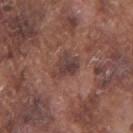biopsy_status: not biopsied; imaged during a skin examination
patient:
  sex: male
  age_approx: 75
lighting: white-light
image:
  source: total-body photography crop
  field_of_view_mm: 15
site: right upper arm
lesion_size:
  long_diameter_mm_approx: 3.5